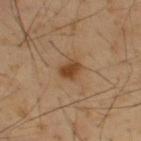workup=total-body-photography surveillance lesion; no biopsy
body site=the upper back
lesion diameter=about 2.5 mm
illumination=cross-polarized
automated lesion analysis=a lesion area of about 4.5 mm², an eccentricity of roughly 0.65, and a symmetry-axis asymmetry near 0.25; a mean CIELAB color near L≈43 a*≈19 b*≈34, a lesion–skin lightness drop of about 11, and a normalized lesion–skin contrast near 9
subject=male, about 40 years old
image=total-body-photography crop, ~15 mm field of view